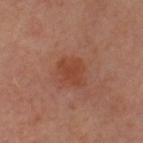Clinical impression: Imaged during a routine full-body skin examination; the lesion was not biopsied and no histopathology is available. Clinical summary: This is a cross-polarized tile. Longest diameter approximately 3.5 mm. A 15 mm close-up tile from a total-body photography series done for melanoma screening. The total-body-photography lesion software estimated an average lesion color of about L≈44 a*≈26 b*≈32 (CIELAB), a lesion–skin lightness drop of about 7, and a normalized lesion–skin contrast near 6.5. The software also gave a border-irregularity index near 3.5/10 and radial color variation of about 0.5. The analysis additionally found a nevus-likeness score of about 30/100 and lesion-presence confidence of about 100/100. From the left upper arm.